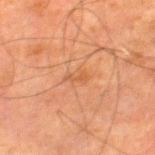follow-up = imaged on a skin check; not biopsied
automated metrics = an average lesion color of about L≈45 a*≈22 b*≈33 (CIELAB) and roughly 5 lightness units darker than nearby skin; a color-variation rating of about 0.5/10 and peripheral color asymmetry of about 0
lesion diameter = ≈2.5 mm
patient = male, about 80 years old
image = 15 mm crop, total-body photography
body site = the leg
lighting = cross-polarized